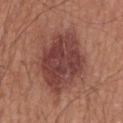<record>
<lighting>white-light</lighting>
<patient>
  <sex>male</sex>
  <age_approx>65</age_approx>
</patient>
<automated_metrics>
  <border_irregularity_0_10>2.5</border_irregularity_0_10>
  <color_variation_0_10>4.5</color_variation_0_10>
  <peripheral_color_asymmetry>1.5</peripheral_color_asymmetry>
  <nevus_likeness_0_100>85</nevus_likeness_0_100>
  <lesion_detection_confidence_0_100>100</lesion_detection_confidence_0_100>
</automated_metrics>
<image>
  <source>total-body photography crop</source>
  <field_of_view_mm>15</field_of_view_mm>
</image>
<site>mid back</site>
<lesion_size>
  <long_diameter_mm_approx>7.5</long_diameter_mm_approx>
</lesion_size>
</record>